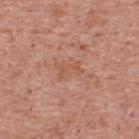Q: Was this lesion biopsied?
A: total-body-photography surveillance lesion; no biopsy
Q: How was the tile lit?
A: white-light illumination
Q: What kind of image is this?
A: 15 mm crop, total-body photography
Q: What is the anatomic site?
A: the upper back
Q: Who is the patient?
A: male, aged approximately 70
Q: What did automated image analysis measure?
A: a lesion area of about 2.5 mm² and a symmetry-axis asymmetry near 0.5; an automated nevus-likeness rating near 0 out of 100 and lesion-presence confidence of about 100/100
Q: Lesion size?
A: ≈2.5 mm